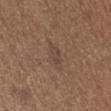No biopsy was performed on this lesion — it was imaged during a full skin examination and was not determined to be concerning. Automated image analysis of the tile measured a lesion–skin lightness drop of about 5 and a normalized lesion–skin contrast near 5. The patient is a male about 65 years old. A 15 mm close-up extracted from a 3D total-body photography capture. The lesion is on the abdomen. Captured under white-light illumination. The lesion's longest dimension is about 3.5 mm.